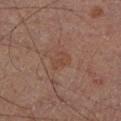biopsy_status: not biopsied; imaged during a skin examination
site: left lower leg
lighting: cross-polarized
automated_metrics:
  border_irregularity_0_10: 4.0
  color_variation_0_10: 1.5
  peripheral_color_asymmetry: 0.5
image:
  source: total-body photography crop
  field_of_view_mm: 15
patient:
  sex: male
  age_approx: 50